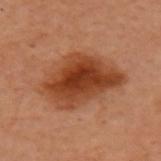| field | value |
|---|---|
| biopsy status | imaged on a skin check; not biopsied |
| patient | female, about 60 years old |
| image source | ~15 mm tile from a whole-body skin photo |
| body site | the left arm |